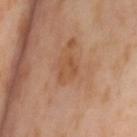Captured during whole-body skin photography for melanoma surveillance; the lesion was not biopsied. The patient is a female about 55 years old. Imaged with cross-polarized lighting. Approximately 2.5 mm at its widest. A roughly 15 mm field-of-view crop from a total-body skin photograph. From the leg.